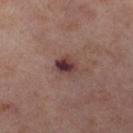Assessment: Recorded during total-body skin imaging; not selected for excision or biopsy. Clinical summary: A female patient, in their mid-50s. The lesion is on the right lower leg. Imaged with cross-polarized lighting. A 15 mm crop from a total-body photograph taken for skin-cancer surveillance. An algorithmic analysis of the crop reported an outline eccentricity of about 0.6 (0 = round, 1 = elongated). It also reported a mean CIELAB color near L≈38 a*≈20 b*≈19, roughly 13 lightness units darker than nearby skin, and a lesion-to-skin contrast of about 10.5 (normalized; higher = more distinct). And it measured a border-irregularity index near 1.5/10 and radial color variation of about 2.5. It also reported an automated nevus-likeness rating near 40 out of 100 and lesion-presence confidence of about 100/100. Measured at roughly 2.5 mm in maximum diameter.